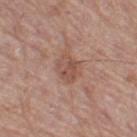{"biopsy_status": "not biopsied; imaged during a skin examination"}